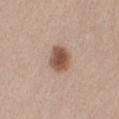| field | value |
|---|---|
| workup | total-body-photography surveillance lesion; no biopsy |
| diameter | about 3 mm |
| body site | the left thigh |
| illumination | white-light illumination |
| image source | 15 mm crop, total-body photography |
| patient | female, aged 23–27 |